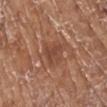| key | value |
|---|---|
| biopsy status | catalogued during a skin exam; not biopsied |
| anatomic site | the right lower leg |
| subject | female, in their mid- to late 70s |
| TBP lesion metrics | a border-irregularity rating of about 4/10 and internal color variation of about 2 on a 0–10 scale; a nevus-likeness score of about 0/100 and a detector confidence of about 90 out of 100 that the crop contains a lesion |
| diameter | ≈3.5 mm |
| image source | ~15 mm crop, total-body skin-cancer survey |
| tile lighting | white-light |